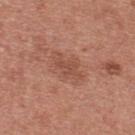This lesion was catalogued during total-body skin photography and was not selected for biopsy.
The total-body-photography lesion software estimated border irregularity of about 5.5 on a 0–10 scale, internal color variation of about 1.5 on a 0–10 scale, and radial color variation of about 0.5.
A lesion tile, about 15 mm wide, cut from a 3D total-body photograph.
Captured under white-light illumination.
A male patient aged around 30.
Approximately 4 mm at its widest.
From the upper back.